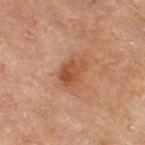Findings:
* image source — ~15 mm tile from a whole-body skin photo
* site — the right thigh
* patient — female, approximately 55 years of age
* illumination — cross-polarized
* automated metrics — a border-irregularity rating of about 3.5/10 and a within-lesion color-variation index near 4.5/10
* diameter — ~4 mm (longest diameter)